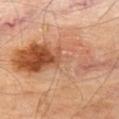Context: Automated image analysis of the tile measured a lesion area of about 45 mm², an eccentricity of roughly 0.95, and a shape-asymmetry score of about 0.35 (0 = symmetric). It also reported a mean CIELAB color near L≈55 a*≈23 b*≈34, a lesion–skin lightness drop of about 12, and a normalized border contrast of about 8. It also reported internal color variation of about 10 on a 0–10 scale and radial color variation of about 4. The lesion's longest dimension is about 15 mm. A region of skin cropped from a whole-body photographic capture, roughly 15 mm wide. The tile uses cross-polarized illumination. The lesion is located on the leg. A male subject in their 70s.